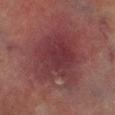Background: The subject is a male aged around 75. The lesion is on the right lower leg. Cropped from a whole-body photographic skin survey; the tile spans about 15 mm.